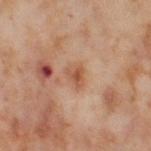Part of a total-body skin-imaging series; this lesion was reviewed on a skin check and was not flagged for biopsy. This image is a 15 mm lesion crop taken from a total-body photograph. Longest diameter approximately 2.5 mm. This is a cross-polarized tile. The subject is a female aged 53–57. From the right thigh.